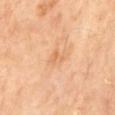| feature | finding |
|---|---|
| biopsy status | catalogued during a skin exam; not biopsied |
| image source | 15 mm crop, total-body photography |
| anatomic site | the back |